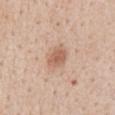  biopsy_status: not biopsied; imaged during a skin examination
  image:
    source: total-body photography crop
    field_of_view_mm: 15
  automated_metrics:
    area_mm2_approx: 6.0
    eccentricity: 0.6
    vs_skin_darker_L: 10.0
    vs_skin_contrast_norm: 7.0
    nevus_likeness_0_100: 90
    lesion_detection_confidence_0_100: 100
  patient:
    sex: male
    age_approx: 60
  lesion_size:
    long_diameter_mm_approx: 3.0
  site: front of the torso
  lighting: white-light